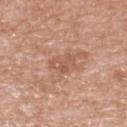The lesion was tiled from a total-body skin photograph and was not biopsied.
About 3 mm across.
From the upper back.
The subject is a male aged 48 to 52.
A close-up tile cropped from a whole-body skin photograph, about 15 mm across.